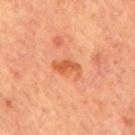Captured during whole-body skin photography for melanoma surveillance; the lesion was not biopsied. The patient is a male approximately 65 years of age. A region of skin cropped from a whole-body photographic capture, roughly 15 mm wide. Located on the mid back.